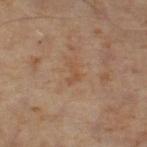Q: Was a biopsy performed?
A: no biopsy performed (imaged during a skin exam)
Q: What is the anatomic site?
A: the left lower leg
Q: How was this image acquired?
A: total-body-photography crop, ~15 mm field of view
Q: How large is the lesion?
A: about 3 mm
Q: What lighting was used for the tile?
A: cross-polarized
Q: Who is the patient?
A: male, approximately 60 years of age
Q: Automated lesion metrics?
A: a lesion area of about 3 mm², a shape eccentricity near 0.85, and two-axis asymmetry of about 0.6; a border-irregularity rating of about 6/10 and internal color variation of about 0 on a 0–10 scale; a nevus-likeness score of about 0/100 and lesion-presence confidence of about 100/100